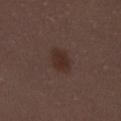Assessment:
Part of a total-body skin-imaging series; this lesion was reviewed on a skin check and was not flagged for biopsy.
Clinical summary:
A 15 mm close-up extracted from a 3D total-body photography capture. Imaged with white-light lighting. Automated image analysis of the tile measured an area of roughly 5.5 mm², an outline eccentricity of about 0.7 (0 = round, 1 = elongated), and a shape-asymmetry score of about 0.2 (0 = symmetric). And it measured about 7 CIELAB-L* units darker than the surrounding skin and a normalized border contrast of about 8. The analysis additionally found border irregularity of about 2 on a 0–10 scale and a within-lesion color-variation index near 2/10. And it measured a nevus-likeness score of about 85/100 and a lesion-detection confidence of about 100/100. The lesion is located on the right upper arm. A male subject aged around 30.